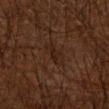A male subject aged around 65. A 15 mm close-up extracted from a 3D total-body photography capture. The lesion is on the right forearm.